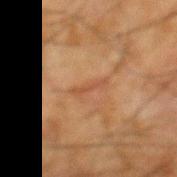Impression:
No biopsy was performed on this lesion — it was imaged during a full skin examination and was not determined to be concerning.
Clinical summary:
The recorded lesion diameter is about 3.5 mm. On the upper back. A 15 mm close-up extracted from a 3D total-body photography capture. A male patient approximately 65 years of age.